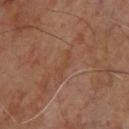| key | value |
|---|---|
| biopsy status | no biopsy performed (imaged during a skin exam) |
| body site | the upper back |
| lesion size | ~3.5 mm (longest diameter) |
| patient | male, approximately 65 years of age |
| illumination | cross-polarized illumination |
| image | ~15 mm tile from a whole-body skin photo |
| automated lesion analysis | a border-irregularity rating of about 7/10, a within-lesion color-variation index near 0/10, and peripheral color asymmetry of about 0; a classifier nevus-likeness of about 0/100 and a lesion-detection confidence of about 65/100 |